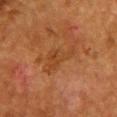No biopsy was performed on this lesion — it was imaged during a full skin examination and was not determined to be concerning. A roughly 15 mm field-of-view crop from a total-body skin photograph. Captured under cross-polarized illumination. Measured at roughly 4.5 mm in maximum diameter. A female patient roughly 50 years of age. Located on the chest. The lesion-visualizer software estimated a shape eccentricity near 0.85 and a shape-asymmetry score of about 0.65 (0 = symmetric). And it measured a border-irregularity rating of about 7/10, a color-variation rating of about 2.5/10, and radial color variation of about 0.5. It also reported an automated nevus-likeness rating near 0 out of 100.